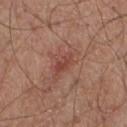Captured during whole-body skin photography for melanoma surveillance; the lesion was not biopsied.
The lesion is on the chest.
A male patient, approximately 55 years of age.
A 15 mm close-up extracted from a 3D total-body photography capture.